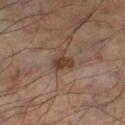Clinical impression:
The lesion was tiled from a total-body skin photograph and was not biopsied.
Background:
A male subject aged 53 to 57. Cropped from a whole-body photographic skin survey; the tile spans about 15 mm. From the left lower leg. Measured at roughly 3 mm in maximum diameter.